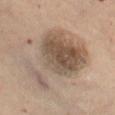biopsy status: no biopsy performed (imaged during a skin exam)
site: the left thigh
patient: female, approximately 60 years of age
tile lighting: cross-polarized illumination
imaging modality: total-body-photography crop, ~15 mm field of view